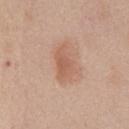Captured during whole-body skin photography for melanoma surveillance; the lesion was not biopsied. A 15 mm close-up tile from a total-body photography series done for melanoma screening. Located on the chest. Longest diameter approximately 4.5 mm. The patient is a female roughly 40 years of age.